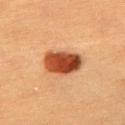The lesion was tiled from a total-body skin photograph and was not biopsied.
About 5.5 mm across.
Automated image analysis of the tile measured a footprint of about 12 mm². It also reported an average lesion color of about L≈41 a*≈27 b*≈35 (CIELAB), a lesion–skin lightness drop of about 18, and a normalized border contrast of about 13.5. The software also gave a detector confidence of about 100 out of 100 that the crop contains a lesion.
A female patient, in their mid-50s.
This image is a 15 mm lesion crop taken from a total-body photograph.
From the chest.